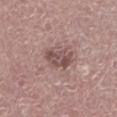Notes:
- notes — catalogued during a skin exam; not biopsied
- diameter — about 4.5 mm
- illumination — white-light
- body site — the left lower leg
- subject — male, aged around 65
- image — 15 mm crop, total-body photography